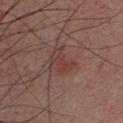workup = imaged on a skin check; not biopsied
anatomic site = the chest
TBP lesion metrics = internal color variation of about 4 on a 0–10 scale and radial color variation of about 1.5; a detector confidence of about 100 out of 100 that the crop contains a lesion
patient = male, about 30 years old
illumination = cross-polarized illumination
imaging modality = 15 mm crop, total-body photography
lesion diameter = about 3 mm